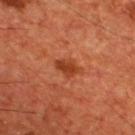This lesion was catalogued during total-body skin photography and was not selected for biopsy. A 15 mm close-up tile from a total-body photography series done for melanoma screening. Measured at roughly 3 mm in maximum diameter. On the front of the torso. The total-body-photography lesion software estimated a symmetry-axis asymmetry near 0.3. The software also gave border irregularity of about 2.5 on a 0–10 scale, a within-lesion color-variation index near 1.5/10, and peripheral color asymmetry of about 0.5. A male subject, aged approximately 55.